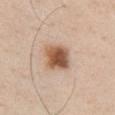Recorded during total-body skin imaging; not selected for excision or biopsy. Located on the chest. A male patient, in their 60s. Automated image analysis of the tile measured a mean CIELAB color near L≈55 a*≈20 b*≈32, roughly 16 lightness units darker than nearby skin, and a normalized border contrast of about 11. It also reported a classifier nevus-likeness of about 100/100 and a detector confidence of about 100 out of 100 that the crop contains a lesion. Imaged with white-light lighting. This image is a 15 mm lesion crop taken from a total-body photograph. Measured at roughly 3.5 mm in maximum diameter.